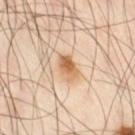No biopsy was performed on this lesion — it was imaged during a full skin examination and was not determined to be concerning. A male patient, aged 48 to 52. From the right thigh. Longest diameter approximately 3 mm. Imaged with cross-polarized lighting. Automated image analysis of the tile measured a lesion area of about 5.5 mm², an eccentricity of roughly 0.75, and a shape-asymmetry score of about 0.2 (0 = symmetric). And it measured an average lesion color of about L≈64 a*≈20 b*≈37 (CIELAB) and a lesion–skin lightness drop of about 12. And it measured an automated nevus-likeness rating near 95 out of 100 and a detector confidence of about 100 out of 100 that the crop contains a lesion. A 15 mm close-up extracted from a 3D total-body photography capture.